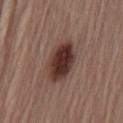Findings:
• workup: no biopsy performed (imaged during a skin exam)
• size: about 5.5 mm
• subject: male, about 55 years old
• image: 15 mm crop, total-body photography
• TBP lesion metrics: two-axis asymmetry of about 0.15; a border-irregularity rating of about 1.5/10, a color-variation rating of about 6/10, and peripheral color asymmetry of about 2
• anatomic site: the abdomen
• lighting: white-light illumination